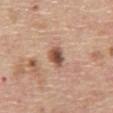notes — catalogued during a skin exam; not biopsied | diameter — ~3 mm (longest diameter) | body site — the abdomen | patient — male, in their 70s | tile lighting — white-light illumination | automated lesion analysis — internal color variation of about 6 on a 0–10 scale and radial color variation of about 2 | imaging modality — ~15 mm crop, total-body skin-cancer survey.